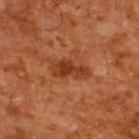Q: Was a biopsy performed?
A: imaged on a skin check; not biopsied
Q: What is the anatomic site?
A: the upper back
Q: What lighting was used for the tile?
A: cross-polarized illumination
Q: Who is the patient?
A: male, roughly 60 years of age
Q: What is the imaging modality?
A: ~15 mm crop, total-body skin-cancer survey
Q: What did automated image analysis measure?
A: an area of roughly 7 mm² and a symmetry-axis asymmetry near 0.3; a lesion color around L≈29 a*≈23 b*≈29 in CIELAB, about 8 CIELAB-L* units darker than the surrounding skin, and a normalized lesion–skin contrast near 8; a within-lesion color-variation index near 3.5/10 and a peripheral color-asymmetry measure near 1; a nevus-likeness score of about 35/100 and lesion-presence confidence of about 100/100